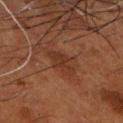Q: Is there a histopathology result?
A: catalogued during a skin exam; not biopsied
Q: What are the patient's age and sex?
A: male, roughly 60 years of age
Q: Automated lesion metrics?
A: an area of roughly 6 mm², an outline eccentricity of about 0.8 (0 = round, 1 = elongated), and a symmetry-axis asymmetry near 0.3
Q: Where on the body is the lesion?
A: the head or neck
Q: What is the lesion's diameter?
A: ≈3.5 mm
Q: Illumination type?
A: cross-polarized illumination
Q: How was this image acquired?
A: ~15 mm crop, total-body skin-cancer survey